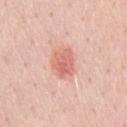{"biopsy_status": "not biopsied; imaged during a skin examination", "site": "chest", "lighting": "white-light", "image": {"source": "total-body photography crop", "field_of_view_mm": 15}, "patient": {"sex": "male", "age_approx": 50}}